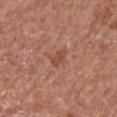The lesion was tiled from a total-body skin photograph and was not biopsied.